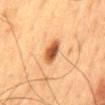biopsy_status: not biopsied; imaged during a skin examination
site: mid back
lighting: cross-polarized
patient:
  sex: male
  age_approx: 60
automated_metrics:
  border_irregularity_0_10: 2.0
  color_variation_0_10: 4.5
lesion_size:
  long_diameter_mm_approx: 3.5
image:
  source: total-body photography crop
  field_of_view_mm: 15